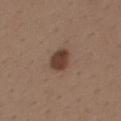{"biopsy_status": "not biopsied; imaged during a skin examination", "automated_metrics": {"area_mm2_approx": 6.0, "shape_asymmetry": 0.15, "nevus_likeness_0_100": 95, "lesion_detection_confidence_0_100": 100}, "image": {"source": "total-body photography crop", "field_of_view_mm": 15}, "site": "upper back", "lighting": "white-light", "patient": {"sex": "male", "age_approx": 40}, "lesion_size": {"long_diameter_mm_approx": 3.0}}